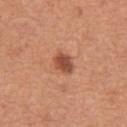Q: Is there a histopathology result?
A: catalogued during a skin exam; not biopsied
Q: Lesion location?
A: the front of the torso
Q: Who is the patient?
A: male, aged 63 to 67
Q: How was this image acquired?
A: ~15 mm tile from a whole-body skin photo
Q: Lesion size?
A: about 3 mm